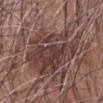| field | value |
|---|---|
| follow-up | no biopsy performed (imaged during a skin exam) |
| location | the left forearm |
| imaging modality | ~15 mm tile from a whole-body skin photo |
| subject | male, aged 58 to 62 |